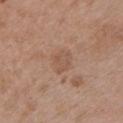Case summary:
* automated lesion analysis · a lesion area of about 5.5 mm², an outline eccentricity of about 0.65 (0 = round, 1 = elongated), and a shape-asymmetry score of about 0.3 (0 = symmetric); an average lesion color of about L≈53 a*≈19 b*≈29 (CIELAB) and roughly 6 lightness units darker than nearby skin; a within-lesion color-variation index near 2/10 and radial color variation of about 0.5; a nevus-likeness score of about 0/100 and lesion-presence confidence of about 100/100
* anatomic site · the left upper arm
* image · ~15 mm crop, total-body skin-cancer survey
* lesion size · about 3 mm
* subject · male, in their 50s